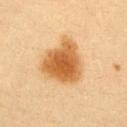Impression: This lesion was catalogued during total-body skin photography and was not selected for biopsy. Clinical summary: A 15 mm close-up tile from a total-body photography series done for melanoma screening. The patient is a female aged 23–27. The lesion is located on the chest. The lesion's longest dimension is about 5.5 mm.